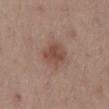<record>
<biopsy_status>not biopsied; imaged during a skin examination</biopsy_status>
<lighting>white-light</lighting>
<patient>
  <sex>male</sex>
  <age_approx>55</age_approx>
</patient>
<lesion_size>
  <long_diameter_mm_approx>3.5</long_diameter_mm_approx>
</lesion_size>
<image>
  <source>total-body photography crop</source>
  <field_of_view_mm>15</field_of_view_mm>
</image>
<site>lower back</site>
</record>